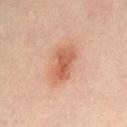Notes:
• biopsy status: total-body-photography surveillance lesion; no biopsy
• subject: female, roughly 40 years of age
• image source: ~15 mm tile from a whole-body skin photo
• diameter: ~5 mm (longest diameter)
• lighting: cross-polarized
• anatomic site: the abdomen